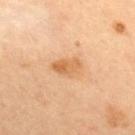<case>
  <biopsy_status>not biopsied; imaged during a skin examination</biopsy_status>
  <image>
    <source>total-body photography crop</source>
    <field_of_view_mm>15</field_of_view_mm>
  </image>
  <site>upper back</site>
  <patient>
    <sex>female</sex>
    <age_approx>35</age_approx>
  </patient>
  <lighting>cross-polarized</lighting>
</case>